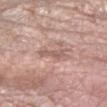<lesion>
<biopsy_status>not biopsied; imaged during a skin examination</biopsy_status>
<lighting>white-light</lighting>
<site>left forearm</site>
<image>
  <source>total-body photography crop</source>
  <field_of_view_mm>15</field_of_view_mm>
</image>
<patient>
  <sex>female</sex>
  <age_approx>70</age_approx>
</patient>
</lesion>